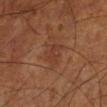<record>
  <site>left lower leg</site>
  <lesion_size>
    <long_diameter_mm_approx>3.0</long_diameter_mm_approx>
  </lesion_size>
  <lighting>cross-polarized</lighting>
  <image>
    <source>total-body photography crop</source>
    <field_of_view_mm>15</field_of_view_mm>
  </image>
  <patient>
    <sex>male</sex>
    <age_approx>70</age_approx>
  </patient>
</record>